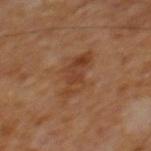The lesion was photographed on a routine skin check and not biopsied; there is no pathology result. A male subject, aged around 65. Cropped from a total-body skin-imaging series; the visible field is about 15 mm. The tile uses cross-polarized illumination. The lesion is located on the mid back.